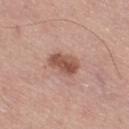The lesion was tiled from a total-body skin photograph and was not biopsied.
A 15 mm close-up tile from a total-body photography series done for melanoma screening.
On the right thigh.
A male patient, approximately 70 years of age.